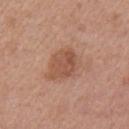The lesion was photographed on a routine skin check and not biopsied; there is no pathology result.
The lesion is located on the left upper arm.
A 15 mm crop from a total-body photograph taken for skin-cancer surveillance.
A female subject approximately 40 years of age.
Automated image analysis of the tile measured a mean CIELAB color near L≈52 a*≈22 b*≈30, about 9 CIELAB-L* units darker than the surrounding skin, and a lesion-to-skin contrast of about 6.5 (normalized; higher = more distinct). And it measured a peripheral color-asymmetry measure near 1. The analysis additionally found an automated nevus-likeness rating near 30 out of 100.